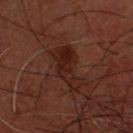workup: no biopsy performed (imaged during a skin exam); body site: the head or neck; acquisition: 15 mm crop, total-body photography; illumination: cross-polarized; subject: male, roughly 60 years of age.